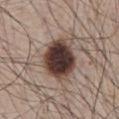– follow-up: catalogued during a skin exam; not biopsied
– automated lesion analysis: an area of roughly 19 mm², an outline eccentricity of about 0.65 (0 = round, 1 = elongated), and a symmetry-axis asymmetry near 0.2; a border-irregularity index near 2/10, a color-variation rating of about 7.5/10, and peripheral color asymmetry of about 1.5; a nevus-likeness score of about 90/100 and a lesion-detection confidence of about 100/100
– location: the chest
– subject: male, in their mid- to late 60s
– acquisition: ~15 mm crop, total-body skin-cancer survey
– lesion size: ~5.5 mm (longest diameter)
– tile lighting: white-light illumination